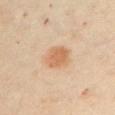Findings:
- workup — total-body-photography surveillance lesion; no biopsy
- illumination — cross-polarized
- subject — female, about 60 years old
- imaging modality — ~15 mm tile from a whole-body skin photo
- image-analysis metrics — a nevus-likeness score of about 100/100
- site — the chest